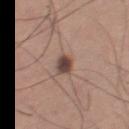This lesion was catalogued during total-body skin photography and was not selected for biopsy. From the right thigh. Measured at roughly 2.5 mm in maximum diameter. A male patient aged 58–62. A 15 mm close-up extracted from a 3D total-body photography capture. An algorithmic analysis of the crop reported about 15 CIELAB-L* units darker than the surrounding skin and a normalized lesion–skin contrast near 11. It also reported a border-irregularity index near 2/10 and a within-lesion color-variation index near 3.5/10. The software also gave a nevus-likeness score of about 90/100. This is a white-light tile.